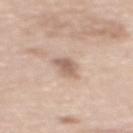Clinical impression:
Captured during whole-body skin photography for melanoma surveillance; the lesion was not biopsied.
Context:
A female patient aged around 60. Approximately 3 mm at its widest. From the back. A 15 mm close-up tile from a total-body photography series done for melanoma screening.